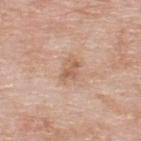The lesion was tiled from a total-body skin photograph and was not biopsied.
The lesion is located on the upper back.
Approximately 3.5 mm at its widest.
The tile uses white-light illumination.
A region of skin cropped from a whole-body photographic capture, roughly 15 mm wide.
A male patient, aged around 60.